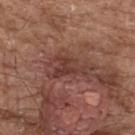This lesion was catalogued during total-body skin photography and was not selected for biopsy. On the upper back. Approximately 4.5 mm at its widest. The subject is a male roughly 75 years of age. A roughly 15 mm field-of-view crop from a total-body skin photograph.